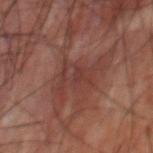Assessment: Imaged during a routine full-body skin examination; the lesion was not biopsied and no histopathology is available. Clinical summary: An algorithmic analysis of the crop reported a footprint of about 9 mm² and an outline eccentricity of about 0.8 (0 = round, 1 = elongated). The analysis additionally found a border-irregularity index near 8/10, internal color variation of about 3.5 on a 0–10 scale, and a peripheral color-asymmetry measure near 1. A male patient in their 60s. Captured under cross-polarized illumination. This image is a 15 mm lesion crop taken from a total-body photograph. On the upper back.